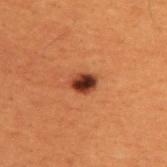Assessment:
Recorded during total-body skin imaging; not selected for excision or biopsy.
Image and clinical context:
From the upper back. Captured under cross-polarized illumination. Longest diameter approximately 2.5 mm. A male patient approximately 50 years of age. A 15 mm crop from a total-body photograph taken for skin-cancer surveillance.